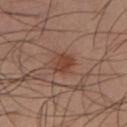Imaged during a routine full-body skin examination; the lesion was not biopsied and no histopathology is available.
A region of skin cropped from a whole-body photographic capture, roughly 15 mm wide.
From the left lower leg.
A male subject aged approximately 40.